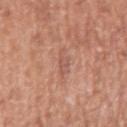A roughly 15 mm field-of-view crop from a total-body skin photograph.
A male subject aged 73–77.
Captured under white-light illumination.
The recorded lesion diameter is about 4 mm.
Automated tile analysis of the lesion measured a lesion area of about 4.5 mm², an outline eccentricity of about 0.95 (0 = round, 1 = elongated), and two-axis asymmetry of about 0.35. And it measured an average lesion color of about L≈56 a*≈23 b*≈28 (CIELAB) and a lesion–skin lightness drop of about 6.
The lesion is on the right upper arm.